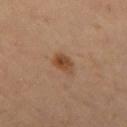Assessment:
Part of a total-body skin-imaging series; this lesion was reviewed on a skin check and was not flagged for biopsy.
Image and clinical context:
A female subject, in their mid-30s. A roughly 15 mm field-of-view crop from a total-body skin photograph. On the left thigh. Longest diameter approximately 3 mm.